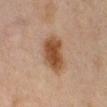Case summary:
- follow-up — no biopsy performed (imaged during a skin exam)
- subject — female, aged approximately 70
- illumination — cross-polarized
- acquisition — ~15 mm tile from a whole-body skin photo
- anatomic site — the right lower leg
- lesion size — about 5 mm